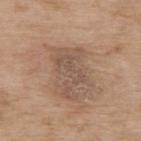Clinical impression:
Captured during whole-body skin photography for melanoma surveillance; the lesion was not biopsied.
Acquisition and patient details:
A female subject aged 73–77. The total-body-photography lesion software estimated an area of roughly 15 mm², an eccentricity of roughly 0.85, and a symmetry-axis asymmetry near 0.4. The software also gave a mean CIELAB color near L≈53 a*≈16 b*≈27 and roughly 8 lightness units darker than nearby skin. A close-up tile cropped from a whole-body skin photograph, about 15 mm across. Located on the back. This is a white-light tile. Approximately 6 mm at its widest.